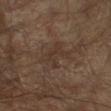Assessment:
Captured during whole-body skin photography for melanoma surveillance; the lesion was not biopsied.
Acquisition and patient details:
Automated image analysis of the tile measured a shape eccentricity near 0.7. The software also gave a mean CIELAB color near L≈32 a*≈14 b*≈22 and roughly 5 lightness units darker than nearby skin. The analysis additionally found a classifier nevus-likeness of about 0/100 and lesion-presence confidence of about 95/100. This is a cross-polarized tile. About 4 mm across. A male subject, aged 63 to 67. On the right forearm. A close-up tile cropped from a whole-body skin photograph, about 15 mm across.